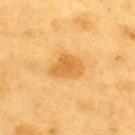The subject is a male in their 60s.
The lesion is located on the upper back.
The recorded lesion diameter is about 4 mm.
A region of skin cropped from a whole-body photographic capture, roughly 15 mm wide.
This is a cross-polarized tile.